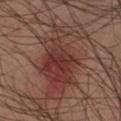Clinical impression: Imaged during a routine full-body skin examination; the lesion was not biopsied and no histopathology is available. Image and clinical context: On the right forearm. Imaged with cross-polarized lighting. A male patient roughly 40 years of age. Longest diameter approximately 9 mm. A region of skin cropped from a whole-body photographic capture, roughly 15 mm wide.